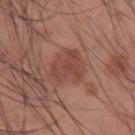{"biopsy_status": "not biopsied; imaged during a skin examination", "lighting": "white-light", "patient": {"sex": "male", "age_approx": 35}, "lesion_size": {"long_diameter_mm_approx": 5.0}, "automated_metrics": {"area_mm2_approx": 12.0, "shape_asymmetry": 0.3, "border_irregularity_0_10": 3.5, "color_variation_0_10": 2.0, "nevus_likeness_0_100": 40, "lesion_detection_confidence_0_100": 100}, "site": "left upper arm", "image": {"source": "total-body photography crop", "field_of_view_mm": 15}}